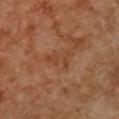The lesion was photographed on a routine skin check and not biopsied; there is no pathology result.
The recorded lesion diameter is about 3.5 mm.
A female patient aged 58 to 62.
Located on the front of the torso.
This is a cross-polarized tile.
A 15 mm crop from a total-body photograph taken for skin-cancer surveillance.